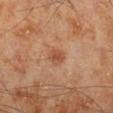{"biopsy_status": "not biopsied; imaged during a skin examination", "site": "right lower leg", "image": {"source": "total-body photography crop", "field_of_view_mm": 15}, "patient": {"sex": "male", "age_approx": 70}}